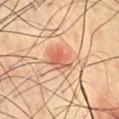<record>
  <site>chest</site>
  <lighting>cross-polarized</lighting>
  <lesion_size>
    <long_diameter_mm_approx>3.0</long_diameter_mm_approx>
  </lesion_size>
  <patient>
    <sex>male</sex>
    <age_approx>70</age_approx>
  </patient>
  <image>
    <source>total-body photography crop</source>
    <field_of_view_mm>15</field_of_view_mm>
  </image>
</record>